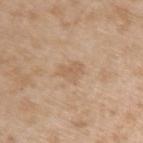Q: How was this image acquired?
A: ~15 mm tile from a whole-body skin photo
Q: What is the anatomic site?
A: the left upper arm
Q: Patient demographics?
A: male, approximately 45 years of age